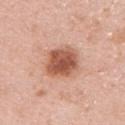From the right upper arm. A female subject, aged approximately 50. Captured under white-light illumination. A roughly 15 mm field-of-view crop from a total-body skin photograph.